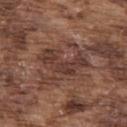Q: Was this lesion biopsied?
A: no biopsy performed (imaged during a skin exam)